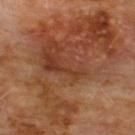Assessment:
Captured during whole-body skin photography for melanoma surveillance; the lesion was not biopsied.
Acquisition and patient details:
Located on the chest. The patient is a male in their 60s. A roughly 15 mm field-of-view crop from a total-body skin photograph.